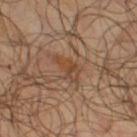<case>
  <biopsy_status>not biopsied; imaged during a skin examination</biopsy_status>
</case>